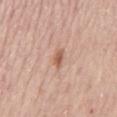Q: Is there a histopathology result?
A: catalogued during a skin exam; not biopsied
Q: What kind of image is this?
A: total-body-photography crop, ~15 mm field of view
Q: Illumination type?
A: white-light illumination
Q: What is the anatomic site?
A: the mid back
Q: What is the lesion's diameter?
A: about 2.5 mm
Q: Who is the patient?
A: female, aged approximately 65